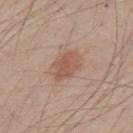The lesion was photographed on a routine skin check and not biopsied; there is no pathology result. A 15 mm crop from a total-body photograph taken for skin-cancer surveillance. The lesion's longest dimension is about 4 mm. A male subject approximately 40 years of age. Automated image analysis of the tile measured a footprint of about 8.5 mm² and two-axis asymmetry of about 0.2. The tile uses white-light illumination. From the mid back.